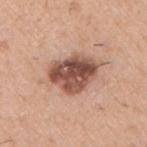Q: Was this lesion biopsied?
A: catalogued during a skin exam; not biopsied
Q: How large is the lesion?
A: ~6 mm (longest diameter)
Q: Patient demographics?
A: male, in their 40s
Q: What kind of image is this?
A: total-body-photography crop, ~15 mm field of view
Q: What did automated image analysis measure?
A: roughly 17 lightness units darker than nearby skin; border irregularity of about 2.5 on a 0–10 scale, a color-variation rating of about 7/10, and radial color variation of about 2.5
Q: Illumination type?
A: white-light illumination
Q: Lesion location?
A: the right upper arm